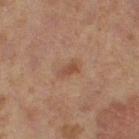Assessment:
This lesion was catalogued during total-body skin photography and was not selected for biopsy.
Context:
A female subject, approximately 60 years of age. A roughly 15 mm field-of-view crop from a total-body skin photograph. Located on the right thigh.